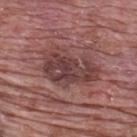Assessment:
Captured during whole-body skin photography for melanoma surveillance; the lesion was not biopsied.
Context:
Imaged with white-light lighting. Located on the back. The subject is a male approximately 70 years of age. Cropped from a whole-body photographic skin survey; the tile spans about 15 mm.